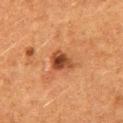The lesion was photographed on a routine skin check and not biopsied; there is no pathology result. Longest diameter approximately 3 mm. A lesion tile, about 15 mm wide, cut from a 3D total-body photograph. From the left thigh. The patient is a female aged approximately 50. Captured under cross-polarized illumination.